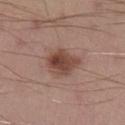| key | value |
|---|---|
| follow-up | catalogued during a skin exam; not biopsied |
| body site | the left lower leg |
| subject | male, roughly 30 years of age |
| image source | ~15 mm tile from a whole-body skin photo |